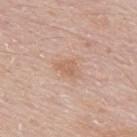Part of a total-body skin-imaging series; this lesion was reviewed on a skin check and was not flagged for biopsy. The patient is a male aged approximately 50. A 15 mm close-up extracted from a 3D total-body photography capture. The lesion is located on the mid back.